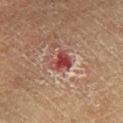Findings:
– follow-up · catalogued during a skin exam; not biopsied
– subject · female, approximately 50 years of age
– tile lighting · cross-polarized
– image source · 15 mm crop, total-body photography
– diameter · ≈3 mm
– location · the right lower leg